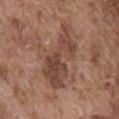Impression: No biopsy was performed on this lesion — it was imaged during a full skin examination and was not determined to be concerning. Background: The patient is a male about 75 years old. The lesion is on the abdomen. The lesion-visualizer software estimated about 10 CIELAB-L* units darker than the surrounding skin and a lesion-to-skin contrast of about 7.5 (normalized; higher = more distinct). The analysis additionally found a classifier nevus-likeness of about 5/100 and a lesion-detection confidence of about 95/100. Approximately 8.5 mm at its widest. Cropped from a whole-body photographic skin survey; the tile spans about 15 mm.